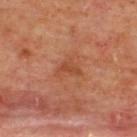Imaged during a routine full-body skin examination; the lesion was not biopsied and no histopathology is available. From the upper back. A roughly 15 mm field-of-view crop from a total-body skin photograph. Captured under cross-polarized illumination. The lesion's longest dimension is about 3 mm. The total-body-photography lesion software estimated an area of roughly 4 mm² and a symmetry-axis asymmetry near 0.55. The analysis additionally found border irregularity of about 5 on a 0–10 scale, a within-lesion color-variation index near 1.5/10, and a peripheral color-asymmetry measure near 0.5. A male subject roughly 65 years of age.